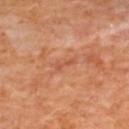Case summary:
• follow-up · catalogued during a skin exam; not biopsied
• image · ~15 mm tile from a whole-body skin photo
• image-analysis metrics · an area of roughly 2 mm² and a shape-asymmetry score of about 0.35 (0 = symmetric)
• body site · the back
• lesion size · about 2.5 mm
• subject · female, aged 48 to 52
• lighting · cross-polarized illumination